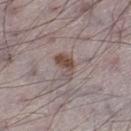Background:
Approximately 3.5 mm at its widest. The patient is a male aged 68–72. Cropped from a whole-body photographic skin survey; the tile spans about 15 mm. The lesion-visualizer software estimated a lesion area of about 6 mm², an eccentricity of roughly 0.8, and two-axis asymmetry of about 0.35. It also reported a lesion–skin lightness drop of about 10 and a normalized lesion–skin contrast near 8. The analysis additionally found a border-irregularity rating of about 3.5/10 and a peripheral color-asymmetry measure near 2.5. Located on the leg. Captured under white-light illumination.